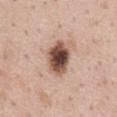Findings:
* biopsy status — catalogued during a skin exam; not biopsied